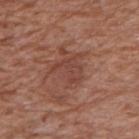Part of a total-body skin-imaging series; this lesion was reviewed on a skin check and was not flagged for biopsy. The lesion's longest dimension is about 3.5 mm. A close-up tile cropped from a whole-body skin photograph, about 15 mm across. Located on the right upper arm. The patient is a male approximately 70 years of age. The tile uses white-light illumination. Automated image analysis of the tile measured a mean CIELAB color near L≈43 a*≈23 b*≈26, about 6 CIELAB-L* units darker than the surrounding skin, and a lesion-to-skin contrast of about 5 (normalized; higher = more distinct). It also reported a color-variation rating of about 1.5/10. The software also gave a classifier nevus-likeness of about 0/100 and a detector confidence of about 95 out of 100 that the crop contains a lesion.